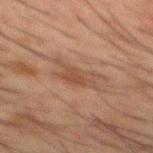The lesion was photographed on a routine skin check and not biopsied; there is no pathology result. Automated tile analysis of the lesion measured a lesion color around L≈37 a*≈16 b*≈25 in CIELAB and about 6 CIELAB-L* units darker than the surrounding skin. And it measured an automated nevus-likeness rating near 0 out of 100 and a lesion-detection confidence of about 100/100. On the mid back. A roughly 15 mm field-of-view crop from a total-body skin photograph. The tile uses cross-polarized illumination. A male patient, aged approximately 50.